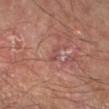{
  "biopsy_status": "not biopsied; imaged during a skin examination",
  "lesion_size": {
    "long_diameter_mm_approx": 2.5
  },
  "lighting": "cross-polarized",
  "image": {
    "source": "total-body photography crop",
    "field_of_view_mm": 15
  },
  "automated_metrics": {
    "area_mm2_approx": 2.5,
    "eccentricity": 0.9,
    "shape_asymmetry": 0.25,
    "nevus_likeness_0_100": 0,
    "lesion_detection_confidence_0_100": 65
  },
  "patient": {
    "sex": "male",
    "age_approx": 65
  },
  "site": "right forearm"
}